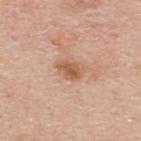Clinical impression:
No biopsy was performed on this lesion — it was imaged during a full skin examination and was not determined to be concerning.
Image and clinical context:
The lesion is on the upper back. About 3 mm across. Captured under white-light illumination. A male subject about 35 years old. A region of skin cropped from a whole-body photographic capture, roughly 15 mm wide.